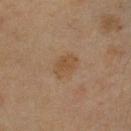notes: total-body-photography surveillance lesion; no biopsy | image source: ~15 mm tile from a whole-body skin photo | lighting: cross-polarized | automated metrics: a footprint of about 6 mm², an eccentricity of roughly 0.65, and two-axis asymmetry of about 0.15 | subject: female, roughly 45 years of age | location: the head or neck | lesion diameter: ≈3 mm.